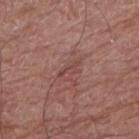No biopsy was performed on this lesion — it was imaged during a full skin examination and was not determined to be concerning. About 2.5 mm across. The patient is a male in their mid- to late 70s. On the right upper arm. A 15 mm close-up tile from a total-body photography series done for melanoma screening.